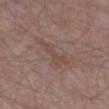Q: Was this lesion biopsied?
A: total-body-photography surveillance lesion; no biopsy
Q: What did automated image analysis measure?
A: an automated nevus-likeness rating near 0 out of 100
Q: What kind of image is this?
A: ~15 mm crop, total-body skin-cancer survey
Q: Illumination type?
A: white-light illumination
Q: What is the lesion's diameter?
A: ~5 mm (longest diameter)
Q: Who is the patient?
A: male, aged 63 to 67
Q: Where on the body is the lesion?
A: the left thigh